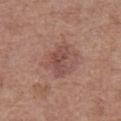{"lighting": "white-light", "automated_metrics": {"area_mm2_approx": 6.0, "eccentricity": 0.8, "shape_asymmetry": 0.4, "cielab_L": 47, "cielab_a": 22, "cielab_b": 24, "vs_skin_darker_L": 8.0, "vs_skin_contrast_norm": 6.5, "border_irregularity_0_10": 4.5, "color_variation_0_10": 2.5, "peripheral_color_asymmetry": 0.5}, "image": {"source": "total-body photography crop", "field_of_view_mm": 15}, "lesion_size": {"long_diameter_mm_approx": 3.5}, "patient": {"sex": "female", "age_approx": 65}, "site": "left thigh"}